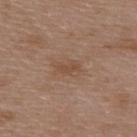Assessment: Captured during whole-body skin photography for melanoma surveillance; the lesion was not biopsied. Context: The lesion is on the upper back. The subject is a male aged 48 to 52. The recorded lesion diameter is about 2.5 mm. The tile uses white-light illumination. This image is a 15 mm lesion crop taken from a total-body photograph.